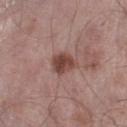Q: Lesion size?
A: ~3 mm (longest diameter)
Q: Who is the patient?
A: male, aged approximately 55
Q: What kind of image is this?
A: ~15 mm tile from a whole-body skin photo
Q: What is the anatomic site?
A: the left lower leg
Q: What lighting was used for the tile?
A: white-light illumination